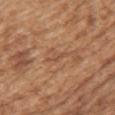Measured at roughly 3 mm in maximum diameter. Located on the upper back. This is a white-light tile. A 15 mm close-up extracted from a 3D total-body photography capture. The patient is a female aged 28 to 32.